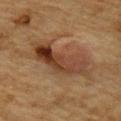Recorded during total-body skin imaging; not selected for excision or biopsy. A close-up tile cropped from a whole-body skin photograph, about 15 mm across. The patient is a male aged approximately 85 or older. From the mid back. The total-body-photography lesion software estimated an area of roughly 20 mm², an outline eccentricity of about 0.95 (0 = round, 1 = elongated), and a shape-asymmetry score of about 0.4 (0 = symmetric). The software also gave a mean CIELAB color near L≈35 a*≈18 b*≈27, roughly 10 lightness units darker than nearby skin, and a normalized border contrast of about 9. The software also gave a border-irregularity rating of about 5.5/10, a within-lesion color-variation index near 9/10, and peripheral color asymmetry of about 4.